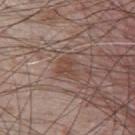Q: Is there a histopathology result?
A: no biopsy performed (imaged during a skin exam)
Q: Lesion location?
A: the chest
Q: What lighting was used for the tile?
A: white-light illumination
Q: Automated lesion metrics?
A: a lesion area of about 5 mm², an outline eccentricity of about 0.4 (0 = round, 1 = elongated), and a shape-asymmetry score of about 0.3 (0 = symmetric); a mean CIELAB color near L≈45 a*≈18 b*≈24 and about 6 CIELAB-L* units darker than the surrounding skin; border irregularity of about 3 on a 0–10 scale, internal color variation of about 1.5 on a 0–10 scale, and radial color variation of about 0.5; a classifier nevus-likeness of about 5/100 and a lesion-detection confidence of about 95/100
Q: What kind of image is this?
A: total-body-photography crop, ~15 mm field of view
Q: Patient demographics?
A: male, approximately 75 years of age
Q: What is the lesion's diameter?
A: ≈2.5 mm